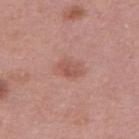A female patient aged around 50. Approximately 3 mm at its widest. This is a white-light tile. Located on the back. A lesion tile, about 15 mm wide, cut from a 3D total-body photograph.